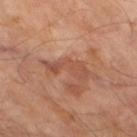  biopsy_status: not biopsied; imaged during a skin examination
  patient:
    sex: male
    age_approx: 70
  image:
    source: total-body photography crop
    field_of_view_mm: 15
  lesion_size:
    long_diameter_mm_approx: 5.5
  site: right thigh